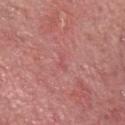Q: Is there a histopathology result?
A: catalogued during a skin exam; not biopsied
Q: Patient demographics?
A: male, approximately 70 years of age
Q: How was this image acquired?
A: ~15 mm tile from a whole-body skin photo
Q: Automated lesion metrics?
A: an area of roughly 2 mm², an eccentricity of roughly 0.8, and two-axis asymmetry of about 0.55
Q: Lesion size?
A: ≈2 mm
Q: What is the anatomic site?
A: the head or neck